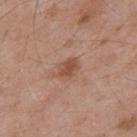Q: Was this lesion biopsied?
A: catalogued during a skin exam; not biopsied
Q: What is the lesion's diameter?
A: ~2.5 mm (longest diameter)
Q: How was this image acquired?
A: ~15 mm tile from a whole-body skin photo
Q: Lesion location?
A: the mid back
Q: Automated lesion metrics?
A: a within-lesion color-variation index near 1.5/10
Q: Patient demographics?
A: male, aged approximately 55